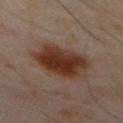Recorded during total-body skin imaging; not selected for excision or biopsy.
A 15 mm crop from a total-body photograph taken for skin-cancer surveillance.
The lesion is located on the mid back.
A male patient aged 68 to 72.
Measured at roughly 5 mm in maximum diameter.